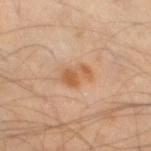| field | value |
|---|---|
| workup | imaged on a skin check; not biopsied |
| subject | male, aged 43–47 |
| anatomic site | the leg |
| image source | total-body-photography crop, ~15 mm field of view |
| lesion size | ~3.5 mm (longest diameter) |
| illumination | cross-polarized |